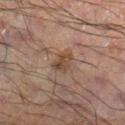Case summary:
- follow-up — total-body-photography surveillance lesion; no biopsy
- size — ≈2.5 mm
- illumination — cross-polarized
- TBP lesion metrics — a lesion area of about 4 mm² and two-axis asymmetry of about 0.3
- imaging modality — total-body-photography crop, ~15 mm field of view
- anatomic site — the right lower leg
- patient — male, roughly 55 years of age